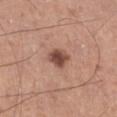This lesion was catalogued during total-body skin photography and was not selected for biopsy.